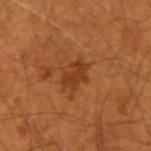follow-up = imaged on a skin check; not biopsied
imaging modality = ~15 mm tile from a whole-body skin photo
automated lesion analysis = a shape eccentricity near 0.75 and a symmetry-axis asymmetry near 0.3; a lesion color around L≈35 a*≈24 b*≈35 in CIELAB and roughly 7 lightness units darker than nearby skin; a classifier nevus-likeness of about 0/100 and a lesion-detection confidence of about 100/100
size = ≈3.5 mm
patient = male, in their mid- to late 50s
anatomic site = the right upper arm